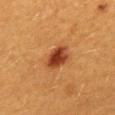automated_metrics:
  vs_skin_darker_L: 13.0
  vs_skin_contrast_norm: 11.0
  border_irregularity_0_10: 2.0
  color_variation_0_10: 4.5
  nevus_likeness_0_100: 100
  lesion_detection_confidence_0_100: 100
lighting: cross-polarized
image:
  source: total-body photography crop
  field_of_view_mm: 15
lesion_size:
  long_diameter_mm_approx: 3.5
site: mid back
patient:
  sex: female
  age_approx: 40